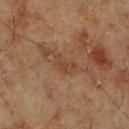| field | value |
|---|---|
| workup | no biopsy performed (imaged during a skin exam) |
| anatomic site | the right lower leg |
| imaging modality | ~15 mm tile from a whole-body skin photo |
| patient | male, approximately 70 years of age |